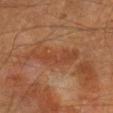Imaged during a routine full-body skin examination; the lesion was not biopsied and no histopathology is available. The tile uses cross-polarized illumination. The lesion-visualizer software estimated a lesion color around L≈38 a*≈23 b*≈30 in CIELAB, about 6 CIELAB-L* units darker than the surrounding skin, and a normalized lesion–skin contrast near 5.5. The analysis additionally found an automated nevus-likeness rating near 0 out of 100. A male subject approximately 65 years of age. The lesion's longest dimension is about 6 mm. Cropped from a whole-body photographic skin survey; the tile spans about 15 mm. On the right lower leg.